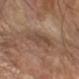workup: no biopsy performed (imaged during a skin exam)
anatomic site: the right forearm
imaging modality: ~15 mm crop, total-body skin-cancer survey